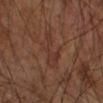Part of a total-body skin-imaging series; this lesion was reviewed on a skin check and was not flagged for biopsy.
The lesion is on the arm.
Cropped from a total-body skin-imaging series; the visible field is about 15 mm.
A male subject, aged around 65.
Longest diameter approximately 4.5 mm.